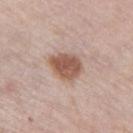Recorded during total-body skin imaging; not selected for excision or biopsy. Imaged with white-light lighting. The lesion's longest dimension is about 4 mm. This image is a 15 mm lesion crop taken from a total-body photograph. The total-body-photography lesion software estimated a lesion area of about 10 mm², a shape eccentricity near 0.6, and a symmetry-axis asymmetry near 0.2. The analysis additionally found a mean CIELAB color near L≈55 a*≈20 b*≈27, a lesion–skin lightness drop of about 14, and a normalized border contrast of about 9.5. The analysis additionally found a border-irregularity index near 2/10, a within-lesion color-variation index near 3/10, and peripheral color asymmetry of about 1. Located on the left thigh. A female patient, aged 58 to 62.